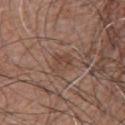Recorded during total-body skin imaging; not selected for excision or biopsy.
A 15 mm close-up tile from a total-body photography series done for melanoma screening.
The subject is a male approximately 65 years of age.
Approximately 3 mm at its widest.
Captured under white-light illumination.
The lesion-visualizer software estimated an eccentricity of roughly 0.75 and two-axis asymmetry of about 0.4. And it measured a color-variation rating of about 3/10.
Located on the chest.